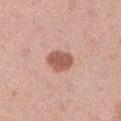The lesion was tiled from a total-body skin photograph and was not biopsied.
The patient is a male approximately 45 years of age.
This is a white-light tile.
This image is a 15 mm lesion crop taken from a total-body photograph.
Located on the left upper arm.
Automated image analysis of the tile measured a border-irregularity rating of about 1.5/10 and internal color variation of about 2.5 on a 0–10 scale.
Approximately 3.5 mm at its widest.